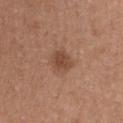Q: What is the anatomic site?
A: the front of the torso
Q: What lighting was used for the tile?
A: white-light
Q: What is the imaging modality?
A: ~15 mm crop, total-body skin-cancer survey
Q: What are the patient's age and sex?
A: female, aged 38–42